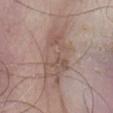site: abdomen
automated_metrics:
  eccentricity: 0.9
  cielab_L: 54
  cielab_a: 16
  cielab_b: 23
  vs_skin_darker_L: 8.0
  vs_skin_contrast_norm: 6.0
  border_irregularity_0_10: 5.0
  color_variation_0_10: 4.5
  peripheral_color_asymmetry: 1.5
  nevus_likeness_0_100: 0
  lesion_detection_confidence_0_100: 60
image:
  source: total-body photography crop
  field_of_view_mm: 15
patient:
  sex: male
  age_approx: 70
lesion_size:
  long_diameter_mm_approx: 8.0